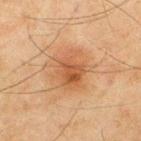The lesion was photographed on a routine skin check and not biopsied; there is no pathology result. This is a cross-polarized tile. A male subject aged approximately 45. The lesion is on the back. Cropped from a total-body skin-imaging series; the visible field is about 15 mm.